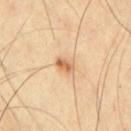lighting = cross-polarized illumination | patient = male, aged around 65 | imaging modality = ~15 mm tile from a whole-body skin photo | automated metrics = a shape eccentricity near 0.85 and a symmetry-axis asymmetry near 0.2; a lesion color around L≈64 a*≈22 b*≈39 in CIELAB; a nevus-likeness score of about 80/100 and a lesion-detection confidence of about 100/100 | site = the mid back.